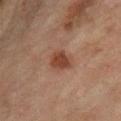<case>
<biopsy_status>not biopsied; imaged during a skin examination</biopsy_status>
<patient>
  <sex>female</sex>
  <age_approx>55</age_approx>
</patient>
<site>leg</site>
<automated_metrics>
  <area_mm2_approx>6.0</area_mm2_approx>
  <eccentricity>0.65</eccentricity>
  <shape_asymmetry>0.25</shape_asymmetry>
  <cielab_L>37</cielab_L>
  <cielab_a>20</cielab_a>
  <cielab_b>26</cielab_b>
  <border_irregularity_0_10>2.0</border_irregularity_0_10>
  <peripheral_color_asymmetry>0.5</peripheral_color_asymmetry>
</automated_metrics>
<lesion_size>
  <long_diameter_mm_approx>3.0</long_diameter_mm_approx>
</lesion_size>
<image>
  <source>total-body photography crop</source>
  <field_of_view_mm>15</field_of_view_mm>
</image>
</case>